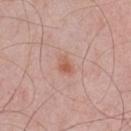Longest diameter approximately 2.5 mm. This image is a 15 mm lesion crop taken from a total-body photograph. The lesion is located on the chest. An algorithmic analysis of the crop reported a footprint of about 4 mm², a shape eccentricity near 0.75, and a symmetry-axis asymmetry near 0.3. The software also gave an average lesion color of about L≈58 a*≈24 b*≈29 (CIELAB) and a normalized border contrast of about 6.5. It also reported a nevus-likeness score of about 40/100 and lesion-presence confidence of about 100/100. A male patient in their 60s.